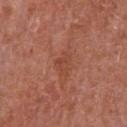| feature | finding |
|---|---|
| follow-up | no biopsy performed (imaged during a skin exam) |
| patient | male, approximately 65 years of age |
| TBP lesion metrics | an eccentricity of roughly 0.8; internal color variation of about 2 on a 0–10 scale and a peripheral color-asymmetry measure near 0.5; a classifier nevus-likeness of about 0/100 |
| anatomic site | the chest |
| lighting | white-light |
| lesion size | ≈3 mm |
| image source | total-body-photography crop, ~15 mm field of view |